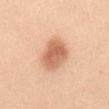Q: Was this lesion biopsied?
A: total-body-photography surveillance lesion; no biopsy
Q: What is the imaging modality?
A: ~15 mm tile from a whole-body skin photo
Q: What is the anatomic site?
A: the mid back
Q: How large is the lesion?
A: about 4.5 mm
Q: What are the patient's age and sex?
A: female, approximately 20 years of age